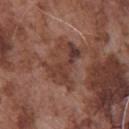Clinical impression:
Recorded during total-body skin imaging; not selected for excision or biopsy.
Context:
A male patient, aged 73–77. Located on the chest. Cropped from a total-body skin-imaging series; the visible field is about 15 mm.